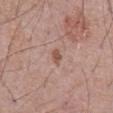  biopsy_status: not biopsied; imaged during a skin examination
  image:
    source: total-body photography crop
    field_of_view_mm: 15
  patient:
    sex: male
    age_approx: 70
  site: chest